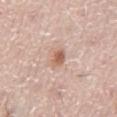{
  "patient": {
    "sex": "male",
    "age_approx": 75
  },
  "site": "front of the torso",
  "lesion_size": {
    "long_diameter_mm_approx": 2.5
  },
  "automated_metrics": {
    "cielab_L": 60,
    "cielab_a": 21,
    "cielab_b": 30,
    "vs_skin_darker_L": 12.0,
    "vs_skin_contrast_norm": 8.0,
    "lesion_detection_confidence_0_100": 100
  },
  "image": {
    "source": "total-body photography crop",
    "field_of_view_mm": 15
  },
  "lighting": "white-light"
}